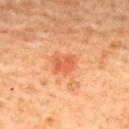A female patient, in their 60s. A 15 mm close-up tile from a total-body photography series done for melanoma screening. The lesion's longest dimension is about 2.5 mm. The total-body-photography lesion software estimated a mean CIELAB color near L≈48 a*≈29 b*≈35 and about 8 CIELAB-L* units darker than the surrounding skin. It also reported a border-irregularity index near 4/10, a color-variation rating of about 1/10, and a peripheral color-asymmetry measure near 0.5. The lesion is located on the upper back. The tile uses cross-polarized illumination.